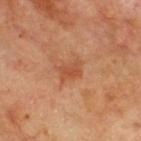No biopsy was performed on this lesion — it was imaged during a full skin examination and was not determined to be concerning. A close-up tile cropped from a whole-body skin photograph, about 15 mm across. A male subject approximately 70 years of age. Captured under cross-polarized illumination. From the upper back.